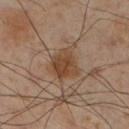Assessment: Recorded during total-body skin imaging; not selected for excision or biopsy. Acquisition and patient details: A lesion tile, about 15 mm wide, cut from a 3D total-body photograph. Approximately 5 mm at its widest. Automated image analysis of the tile measured a footprint of about 12 mm² and a shape eccentricity near 0.65. The analysis additionally found border irregularity of about 4 on a 0–10 scale and internal color variation of about 4 on a 0–10 scale. The lesion is on the right lower leg. This is a cross-polarized tile. The subject is a male approximately 55 years of age.